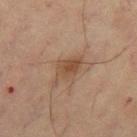Q: Is there a histopathology result?
A: imaged on a skin check; not biopsied
Q: Automated lesion metrics?
A: a lesion color around L≈42 a*≈16 b*≈27 in CIELAB and a normalized lesion–skin contrast near 6.5; a within-lesion color-variation index near 3/10 and a peripheral color-asymmetry measure near 1
Q: What is the imaging modality?
A: ~15 mm crop, total-body skin-cancer survey
Q: Who is the patient?
A: female, about 55 years old
Q: What lighting was used for the tile?
A: cross-polarized
Q: Where on the body is the lesion?
A: the leg